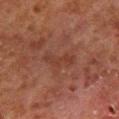Q: Was this lesion biopsied?
A: total-body-photography surveillance lesion; no biopsy
Q: Who is the patient?
A: male, approximately 80 years of age
Q: What is the imaging modality?
A: ~15 mm tile from a whole-body skin photo
Q: Lesion location?
A: the right lower leg